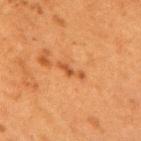Assessment: Part of a total-body skin-imaging series; this lesion was reviewed on a skin check and was not flagged for biopsy. Acquisition and patient details: Located on the upper back. This image is a 15 mm lesion crop taken from a total-body photograph. The total-body-photography lesion software estimated a border-irregularity rating of about 5.5/10 and internal color variation of about 0 on a 0–10 scale. The software also gave a detector confidence of about 100 out of 100 that the crop contains a lesion. Imaged with cross-polarized lighting. A female patient, aged around 50. Longest diameter approximately 3.5 mm.